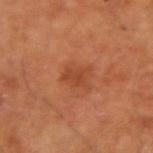Clinical impression:
The lesion was tiled from a total-body skin photograph and was not biopsied.
Context:
The total-body-photography lesion software estimated a border-irregularity index near 4.5/10, a color-variation rating of about 1.5/10, and radial color variation of about 0.5. The patient is a male aged approximately 60. A lesion tile, about 15 mm wide, cut from a 3D total-body photograph. Captured under cross-polarized illumination. Approximately 3 mm at its widest. On the arm.